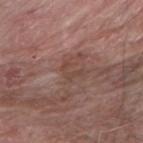site: the right forearm; acquisition: ~15 mm tile from a whole-body skin photo; lighting: white-light; subject: male, aged 73 to 77; lesion diameter: about 2.5 mm.